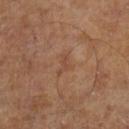This lesion was catalogued during total-body skin photography and was not selected for biopsy. Imaged with cross-polarized lighting. Automated image analysis of the tile measured a lesion–skin lightness drop of about 6. And it measured border irregularity of about 5 on a 0–10 scale, a within-lesion color-variation index near 0/10, and a peripheral color-asymmetry measure near 0. A male subject aged around 65. A roughly 15 mm field-of-view crop from a total-body skin photograph. The lesion's longest dimension is about 2.5 mm.